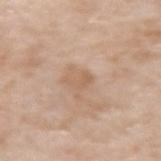follow-up = catalogued during a skin exam; not biopsied | location = the right forearm | subject = female, approximately 70 years of age | image source = total-body-photography crop, ~15 mm field of view.